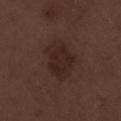The lesion was tiled from a total-body skin photograph and was not biopsied. The tile uses white-light illumination. Automated image analysis of the tile measured an area of roughly 15 mm² and two-axis asymmetry of about 0.25. It also reported a nevus-likeness score of about 40/100 and a detector confidence of about 100 out of 100 that the crop contains a lesion. The patient is a male aged 68 to 72. Cropped from a whole-body photographic skin survey; the tile spans about 15 mm. Longest diameter approximately 5 mm. Located on the right lower leg.